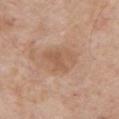workup: imaged on a skin check; not biopsied | location: the chest | patient: male, in their mid- to late 60s | image: ~15 mm tile from a whole-body skin photo.